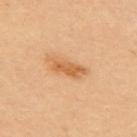{"biopsy_status": "not biopsied; imaged during a skin examination", "image": {"source": "total-body photography crop", "field_of_view_mm": 15}, "patient": {"sex": "female", "age_approx": 35}, "site": "upper back", "automated_metrics": {"area_mm2_approx": 6.0, "eccentricity": 0.9, "shape_asymmetry": 0.25}, "lighting": "cross-polarized"}